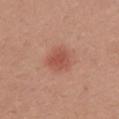Part of a total-body skin-imaging series; this lesion was reviewed on a skin check and was not flagged for biopsy. The lesion is on the mid back. The total-body-photography lesion software estimated a mean CIELAB color near L≈53 a*≈27 b*≈30, a lesion–skin lightness drop of about 9, and a normalized lesion–skin contrast near 6.5. It also reported border irregularity of about 1.5 on a 0–10 scale, internal color variation of about 2.5 on a 0–10 scale, and peripheral color asymmetry of about 1. Imaged with white-light lighting. About 3.5 mm across. A female subject roughly 25 years of age. A lesion tile, about 15 mm wide, cut from a 3D total-body photograph.